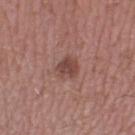biopsy_status: not biopsied; imaged during a skin examination
site: left lower leg
automated_metrics:
  eccentricity: 0.4
  shape_asymmetry: 0.25
  cielab_L: 45
  cielab_a: 21
  cielab_b: 23
  vs_skin_darker_L: 10.0
  vs_skin_contrast_norm: 7.5
patient:
  sex: male
  age_approx: 55
lesion_size:
  long_diameter_mm_approx: 2.5
lighting: white-light
image:
  source: total-body photography crop
  field_of_view_mm: 15